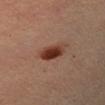Q: Was this lesion biopsied?
A: total-body-photography surveillance lesion; no biopsy
Q: How large is the lesion?
A: ~3.5 mm (longest diameter)
Q: What are the patient's age and sex?
A: female, aged 33 to 37
Q: What kind of image is this?
A: 15 mm crop, total-body photography
Q: Where on the body is the lesion?
A: the left forearm